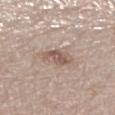The tile uses white-light illumination. Cropped from a total-body skin-imaging series; the visible field is about 15 mm. Automated image analysis of the tile measured a shape eccentricity near 0.8 and a shape-asymmetry score of about 0.2 (0 = symmetric). The recorded lesion diameter is about 3.5 mm. A female subject approximately 55 years of age. On the right lower leg.